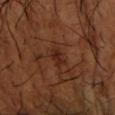Impression: Part of a total-body skin-imaging series; this lesion was reviewed on a skin check and was not flagged for biopsy. Background: Imaged with cross-polarized lighting. The recorded lesion diameter is about 2.5 mm. A male subject, in their mid-60s. On the arm. A 15 mm close-up extracted from a 3D total-body photography capture.